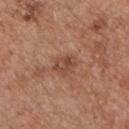{"patient": {"sex": "male", "age_approx": 55}, "automated_metrics": {"vs_skin_darker_L": 8.0}, "image": {"source": "total-body photography crop", "field_of_view_mm": 15}, "site": "chest", "lighting": "white-light"}